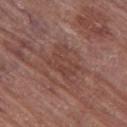diameter — about 4.5 mm | subject — female, roughly 80 years of age | site — the left thigh | image source — ~15 mm tile from a whole-body skin photo | tile lighting — white-light.